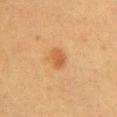Impression: Part of a total-body skin-imaging series; this lesion was reviewed on a skin check and was not flagged for biopsy. Background: Imaged with cross-polarized lighting. The total-body-photography lesion software estimated an area of roughly 4.5 mm². It also reported an average lesion color of about L≈47 a*≈21 b*≈35 (CIELAB), roughly 8 lightness units darker than nearby skin, and a normalized lesion–skin contrast near 6.5. And it measured a border-irregularity index near 2/10, a color-variation rating of about 2/10, and radial color variation of about 1. The software also gave a classifier nevus-likeness of about 90/100. The lesion's longest dimension is about 3 mm. A female subject, aged approximately 40. From the left upper arm. This image is a 15 mm lesion crop taken from a total-body photograph.